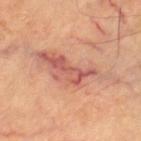Imaged during a routine full-body skin examination; the lesion was not biopsied and no histopathology is available.
Approximately 10.5 mm at its widest.
A roughly 15 mm field-of-view crop from a total-body skin photograph.
An algorithmic analysis of the crop reported an area of roughly 20 mm², a shape eccentricity near 0.95, and a symmetry-axis asymmetry near 0.5. The software also gave a lesion color around L≈59 a*≈25 b*≈29 in CIELAB, roughly 10 lightness units darker than nearby skin, and a lesion-to-skin contrast of about 7 (normalized; higher = more distinct). The software also gave a border-irregularity index near 8/10, a color-variation rating of about 6.5/10, and radial color variation of about 2. The analysis additionally found a lesion-detection confidence of about 85/100.
On the left thigh.
Captured under cross-polarized illumination.
The patient is approximately 65 years of age.